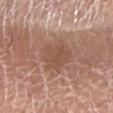follow-up — total-body-photography surveillance lesion; no biopsy
acquisition — ~15 mm tile from a whole-body skin photo
diameter — about 4.5 mm
anatomic site — the arm
patient — male, aged approximately 65
automated lesion analysis — an automated nevus-likeness rating near 55 out of 100